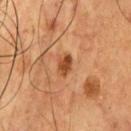• body site · the front of the torso
• image source · 15 mm crop, total-body photography
• patient · male, about 55 years old
• tile lighting · cross-polarized
• automated metrics · a lesion area of about 3.5 mm², an outline eccentricity of about 0.8 (0 = round, 1 = elongated), and two-axis asymmetry of about 0.25; a border-irregularity rating of about 2/10, internal color variation of about 1.5 on a 0–10 scale, and radial color variation of about 0.5